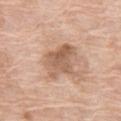subject: female, in their mid-70s
image: ~15 mm crop, total-body skin-cancer survey
lesion size: ~4.5 mm (longest diameter)
tile lighting: white-light illumination
site: the left thigh